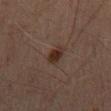Q: Was a biopsy performed?
A: imaged on a skin check; not biopsied
Q: What is the imaging modality?
A: ~15 mm tile from a whole-body skin photo
Q: What is the lesion's diameter?
A: ≈2.5 mm
Q: What are the patient's age and sex?
A: male, in their 70s
Q: Automated lesion metrics?
A: a lesion area of about 3.5 mm²; border irregularity of about 2 on a 0–10 scale and a within-lesion color-variation index near 1.5/10
Q: How was the tile lit?
A: cross-polarized
Q: Where on the body is the lesion?
A: the abdomen